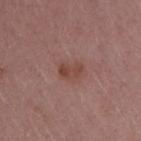This lesion was catalogued during total-body skin photography and was not selected for biopsy.
Longest diameter approximately 2.5 mm.
A female patient in their mid-40s.
The tile uses white-light illumination.
Cropped from a total-body skin-imaging series; the visible field is about 15 mm.
Automated image analysis of the tile measured an area of roughly 4 mm², an eccentricity of roughly 0.8, and a symmetry-axis asymmetry near 0.3. The analysis additionally found a lesion–skin lightness drop of about 8 and a lesion-to-skin contrast of about 7 (normalized; higher = more distinct). It also reported border irregularity of about 3 on a 0–10 scale and peripheral color asymmetry of about 1.
The lesion is located on the arm.